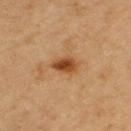Findings:
– automated lesion analysis: a lesion area of about 6.5 mm² and an eccentricity of roughly 0.8; about 11 CIELAB-L* units darker than the surrounding skin and a normalized lesion–skin contrast near 9; a color-variation rating of about 5/10 and a peripheral color-asymmetry measure near 1.5
– image: ~15 mm crop, total-body skin-cancer survey
– subject: female, roughly 70 years of age
– diameter: about 3.5 mm
– lighting: cross-polarized
– body site: the arm